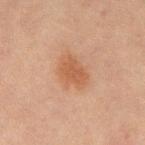Background:
This is a cross-polarized tile. A roughly 15 mm field-of-view crop from a total-body skin photograph. From the mid back. A male patient, about 65 years old.